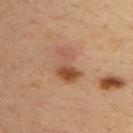The lesion was photographed on a routine skin check and not biopsied; there is no pathology result. A 15 mm close-up tile from a total-body photography series done for melanoma screening. The subject is a male aged approximately 35. On the upper back.